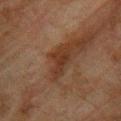Captured during whole-body skin photography for melanoma surveillance; the lesion was not biopsied. Cropped from a total-body skin-imaging series; the visible field is about 15 mm. A male patient aged 73 to 77. From the upper back.